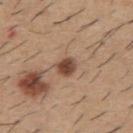lesion size = ≈2.5 mm
automated metrics = a mean CIELAB color near L≈46 a*≈20 b*≈29, roughly 15 lightness units darker than nearby skin, and a normalized border contrast of about 10.5; border irregularity of about 1.5 on a 0–10 scale
body site = the back
patient = male, aged around 60
acquisition = total-body-photography crop, ~15 mm field of view
illumination = white-light